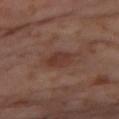No biopsy was performed on this lesion — it was imaged during a full skin examination and was not determined to be concerning. A female patient, about 55 years old. Measured at roughly 3.5 mm in maximum diameter. A roughly 15 mm field-of-view crop from a total-body skin photograph. The tile uses cross-polarized illumination. The lesion is on the right thigh.